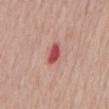Recorded during total-body skin imaging; not selected for excision or biopsy. Measured at roughly 2.5 mm in maximum diameter. Automated image analysis of the tile measured an average lesion color of about L≈52 a*≈34 b*≈25 (CIELAB), a lesion–skin lightness drop of about 14, and a normalized border contrast of about 9.5. Cropped from a total-body skin-imaging series; the visible field is about 15 mm. This is a white-light tile. A female patient, approximately 75 years of age. The lesion is located on the mid back.